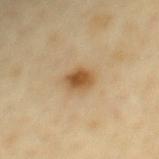This is a cross-polarized tile.
Approximately 3 mm at its widest.
On the chest.
The patient is a male about 65 years old.
Cropped from a total-body skin-imaging series; the visible field is about 15 mm.